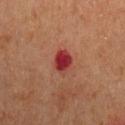This lesion was catalogued during total-body skin photography and was not selected for biopsy.